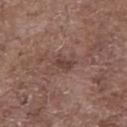Q: Was this lesion biopsied?
A: imaged on a skin check; not biopsied
Q: How was this image acquired?
A: total-body-photography crop, ~15 mm field of view
Q: What is the lesion's diameter?
A: ≈2.5 mm
Q: Automated lesion metrics?
A: an eccentricity of roughly 0.75 and a symmetry-axis asymmetry near 0.25; a lesion color around L≈42 a*≈18 b*≈22 in CIELAB, a lesion–skin lightness drop of about 8, and a lesion-to-skin contrast of about 6.5 (normalized; higher = more distinct); border irregularity of about 2.5 on a 0–10 scale and a within-lesion color-variation index near 2/10
Q: What lighting was used for the tile?
A: white-light illumination
Q: Who is the patient?
A: male, about 70 years old
Q: Lesion location?
A: the chest